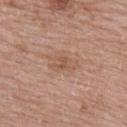workup: no biopsy performed (imaged during a skin exam)
lesion size: about 4.5 mm
illumination: white-light
subject: female, roughly 50 years of age
acquisition: ~15 mm tile from a whole-body skin photo
site: the upper back
TBP lesion metrics: a lesion area of about 11 mm², an eccentricity of roughly 0.75, and a shape-asymmetry score of about 0.35 (0 = symmetric); border irregularity of about 4.5 on a 0–10 scale and a within-lesion color-variation index near 3/10; an automated nevus-likeness rating near 0 out of 100 and a lesion-detection confidence of about 100/100